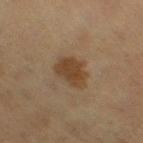* biopsy status · imaged on a skin check; not biopsied